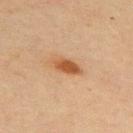Recorded during total-body skin imaging; not selected for excision or biopsy.
A 15 mm close-up extracted from a 3D total-body photography capture.
The lesion is located on the upper back.
The subject is a female aged approximately 45.
An algorithmic analysis of the crop reported a lesion color around L≈48 a*≈21 b*≈34 in CIELAB, a lesion–skin lightness drop of about 11, and a normalized border contrast of about 9. The software also gave a classifier nevus-likeness of about 95/100.
Imaged with cross-polarized lighting.
Approximately 4 mm at its widest.